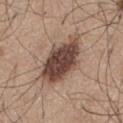Imaged during a routine full-body skin examination; the lesion was not biopsied and no histopathology is available. The lesion's longest dimension is about 7.5 mm. From the chest. Captured under white-light illumination. The subject is a male aged 53–57. A region of skin cropped from a whole-body photographic capture, roughly 15 mm wide. The lesion-visualizer software estimated a border-irregularity index near 2.5/10 and a peripheral color-asymmetry measure near 1.5. The analysis additionally found a classifier nevus-likeness of about 60/100 and a detector confidence of about 100 out of 100 that the crop contains a lesion.